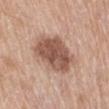Imaged during a routine full-body skin examination; the lesion was not biopsied and no histopathology is available. The lesion is on the left upper arm. Imaged with white-light lighting. A male subject approximately 70 years of age. A roughly 15 mm field-of-view crop from a total-body skin photograph. An algorithmic analysis of the crop reported internal color variation of about 4.5 on a 0–10 scale and a peripheral color-asymmetry measure near 1.5. And it measured a lesion-detection confidence of about 100/100.